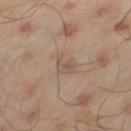Automated tile analysis of the lesion measured a lesion color around L≈52 a*≈15 b*≈25 in CIELAB and a lesion–skin lightness drop of about 7. The analysis additionally found a classifier nevus-likeness of about 0/100 and a lesion-detection confidence of about 100/100.
Cropped from a total-body skin-imaging series; the visible field is about 15 mm.
The lesion is on the leg.
A male subject, roughly 45 years of age.